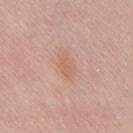Clinical impression: No biopsy was performed on this lesion — it was imaged during a full skin examination and was not determined to be concerning. Image and clinical context: Measured at roughly 3 mm in maximum diameter. The lesion is located on the lower back. The lesion-visualizer software estimated a lesion area of about 4.5 mm², a shape eccentricity near 0.85, and two-axis asymmetry of about 0.25. It also reported border irregularity of about 2.5 on a 0–10 scale and peripheral color asymmetry of about 0.5. Captured under white-light illumination. A 15 mm close-up extracted from a 3D total-body photography capture. The patient is a male about 55 years old.